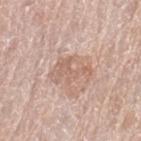Recorded during total-body skin imaging; not selected for excision or biopsy.
A female patient, aged 58–62.
Located on the left thigh.
The tile uses white-light illumination.
A 15 mm crop from a total-body photograph taken for skin-cancer surveillance.